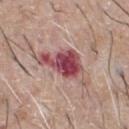  biopsy_status: not biopsied; imaged during a skin examination
  patient:
    sex: male
    age_approx: 65
  site: chest
  image:
    source: total-body photography crop
    field_of_view_mm: 15
  lesion_size:
    long_diameter_mm_approx: 7.0
  automated_metrics:
    cielab_L: 49
    cielab_a: 28
    cielab_b: 19
    vs_skin_contrast_norm: 11.0
  lighting: white-light